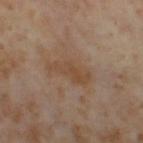The lesion was tiled from a total-body skin photograph and was not biopsied.
A region of skin cropped from a whole-body photographic capture, roughly 15 mm wide.
Measured at roughly 5 mm in maximum diameter.
Automated tile analysis of the lesion measured a footprint of about 8.5 mm², an eccentricity of roughly 0.9, and a symmetry-axis asymmetry near 0.4. And it measured roughly 6 lightness units darker than nearby skin and a normalized lesion–skin contrast near 6.5. The analysis additionally found an automated nevus-likeness rating near 0 out of 100 and a detector confidence of about 100 out of 100 that the crop contains a lesion.
A female patient, roughly 55 years of age.
On the right thigh.